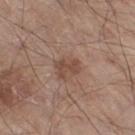Case summary:
• workup · imaged on a skin check; not biopsied
• anatomic site · the leg
• size · about 3 mm
• tile lighting · white-light illumination
• subject · male, aged 78–82
• TBP lesion metrics · border irregularity of about 4 on a 0–10 scale, internal color variation of about 1.5 on a 0–10 scale, and a peripheral color-asymmetry measure near 0.5; a nevus-likeness score of about 25/100 and a detector confidence of about 100 out of 100 that the crop contains a lesion
• image · ~15 mm crop, total-body skin-cancer survey